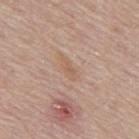Recorded during total-body skin imaging; not selected for excision or biopsy. The lesion is located on the upper back. A region of skin cropped from a whole-body photographic capture, roughly 15 mm wide. A male subject approximately 80 years of age.